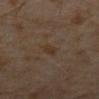Q: Was this lesion biopsied?
A: no biopsy performed (imaged during a skin exam)
Q: What kind of image is this?
A: 15 mm crop, total-body photography
Q: What did automated image analysis measure?
A: an automated nevus-likeness rating near 0 out of 100 and a lesion-detection confidence of about 100/100
Q: Who is the patient?
A: male, aged 63 to 67
Q: Lesion size?
A: ~2.5 mm (longest diameter)